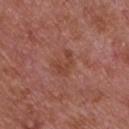Q: Was this lesion biopsied?
A: total-body-photography surveillance lesion; no biopsy
Q: How was the tile lit?
A: white-light
Q: What is the anatomic site?
A: the front of the torso
Q: What is the imaging modality?
A: 15 mm crop, total-body photography
Q: What is the lesion's diameter?
A: ≈3.5 mm
Q: What are the patient's age and sex?
A: male, approximately 65 years of age